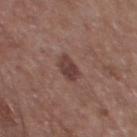Clinical impression:
Recorded during total-body skin imaging; not selected for excision or biopsy.
Context:
Automated image analysis of the tile measured an outline eccentricity of about 0.65 (0 = round, 1 = elongated) and a shape-asymmetry score of about 0.2 (0 = symmetric). It also reported a nevus-likeness score of about 50/100 and lesion-presence confidence of about 100/100. The patient is a male approximately 55 years of age. Cropped from a whole-body photographic skin survey; the tile spans about 15 mm. On the front of the torso. The lesion's longest dimension is about 3 mm.